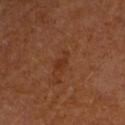Q: Is there a histopathology result?
A: catalogued during a skin exam; not biopsied
Q: How large is the lesion?
A: ≈2.5 mm
Q: Automated lesion metrics?
A: two-axis asymmetry of about 0.4; an automated nevus-likeness rating near 0 out of 100 and a lesion-detection confidence of about 100/100
Q: How was the tile lit?
A: cross-polarized illumination
Q: Patient demographics?
A: female
Q: How was this image acquired?
A: 15 mm crop, total-body photography
Q: Lesion location?
A: the arm